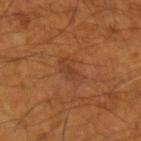Findings:
- image source · ~15 mm tile from a whole-body skin photo
- patient · male, aged around 55
- automated lesion analysis · a symmetry-axis asymmetry near 0.25
- tile lighting · cross-polarized
- site · the left forearm
- lesion diameter · ≈4 mm